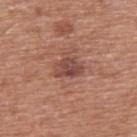The lesion was photographed on a routine skin check and not biopsied; there is no pathology result.
The lesion is on the upper back.
A 15 mm close-up extracted from a 3D total-body photography capture.
Longest diameter approximately 3.5 mm.
A male patient aged 73 to 77.
An algorithmic analysis of the crop reported a footprint of about 6 mm² and a shape eccentricity near 0.75. And it measured a mean CIELAB color near L≈46 a*≈23 b*≈26. The analysis additionally found internal color variation of about 3 on a 0–10 scale and radial color variation of about 1. The software also gave an automated nevus-likeness rating near 20 out of 100 and lesion-presence confidence of about 100/100.